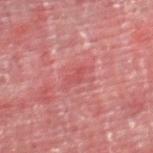biopsy status: no biopsy performed (imaged during a skin exam)
size: ≈2.5 mm
location: the right thigh
TBP lesion metrics: an eccentricity of roughly 0.85 and a shape-asymmetry score of about 0.4 (0 = symmetric); a mean CIELAB color near L≈46 a*≈32 b*≈22, about 6 CIELAB-L* units darker than the surrounding skin, and a lesion-to-skin contrast of about 4.5 (normalized; higher = more distinct); a border-irregularity rating of about 4/10, a within-lesion color-variation index near 0/10, and peripheral color asymmetry of about 0
subject: male, roughly 55 years of age
image source: 15 mm crop, total-body photography